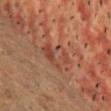Clinical summary:
A lesion tile, about 15 mm wide, cut from a 3D total-body photograph. The patient is a male aged 53 to 57. This is a cross-polarized tile. The lesion is located on the head or neck. Measured at roughly 4 mm in maximum diameter.